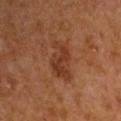workup: catalogued during a skin exam; not biopsied
subject: male, aged 63 to 67
automated metrics: an area of roughly 11 mm², an eccentricity of roughly 0.85, and a shape-asymmetry score of about 0.35 (0 = symmetric); border irregularity of about 4.5 on a 0–10 scale and peripheral color asymmetry of about 1; a classifier nevus-likeness of about 35/100 and lesion-presence confidence of about 100/100
image: ~15 mm tile from a whole-body skin photo
illumination: cross-polarized
diameter: about 5.5 mm
body site: the right upper arm